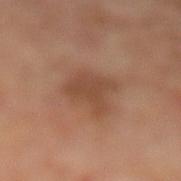- notes: catalogued during a skin exam; not biopsied
- tile lighting: cross-polarized illumination
- diameter: ~4 mm (longest diameter)
- location: the left lower leg
- image source: ~15 mm crop, total-body skin-cancer survey
- patient: male, about 50 years old